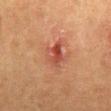Q: Was this lesion biopsied?
A: imaged on a skin check; not biopsied
Q: Automated lesion metrics?
A: border irregularity of about 3.5 on a 0–10 scale, a color-variation rating of about 7/10, and peripheral color asymmetry of about 2
Q: Illumination type?
A: cross-polarized
Q: Where on the body is the lesion?
A: the front of the torso
Q: How large is the lesion?
A: about 3.5 mm
Q: How was this image acquired?
A: ~15 mm crop, total-body skin-cancer survey
Q: Patient demographics?
A: female, in their 50s